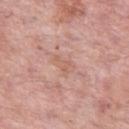The lesion was tiled from a total-body skin photograph and was not biopsied. From the left thigh. The patient is a female about 60 years old. The recorded lesion diameter is about 3 mm. Cropped from a total-body skin-imaging series; the visible field is about 15 mm. The lesion-visualizer software estimated a footprint of about 4 mm² and a shape-asymmetry score of about 0.3 (0 = symmetric). And it measured roughly 6 lightness units darker than nearby skin. And it measured a within-lesion color-variation index near 1.5/10 and radial color variation of about 0.5.